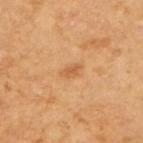  automated_metrics:
    area_mm2_approx: 2.5
    eccentricity: 0.85
    shape_asymmetry: 0.3
    nevus_likeness_0_100: 10
    lesion_detection_confidence_0_100: 100
  site: right upper arm
  lesion_size:
    long_diameter_mm_approx: 2.5
  patient:
    sex: female
    age_approx: 55
  lighting: cross-polarized
  image:
    source: total-body photography crop
    field_of_view_mm: 15